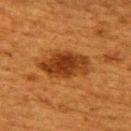Case summary:
- workup — imaged on a skin check; not biopsied
- lighting — cross-polarized illumination
- site — the upper back
- subject — female, in their 50s
- lesion diameter — ≈6 mm
- image source — total-body-photography crop, ~15 mm field of view
- image-analysis metrics — an outline eccentricity of about 0.85 (0 = round, 1 = elongated); border irregularity of about 2 on a 0–10 scale, a color-variation rating of about 4/10, and radial color variation of about 1; a nevus-likeness score of about 90/100 and lesion-presence confidence of about 100/100